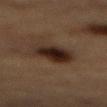<case>
  <biopsy_status>not biopsied; imaged during a skin examination</biopsy_status>
  <image>
    <source>total-body photography crop</source>
    <field_of_view_mm>15</field_of_view_mm>
  </image>
  <lighting>cross-polarized</lighting>
  <patient>
    <sex>male</sex>
    <age_approx>85+</age_approx>
  </patient>
  <lesion_size>
    <long_diameter_mm_approx>4.5</long_diameter_mm_approx>
  </lesion_size>
  <site>back</site>
</case>